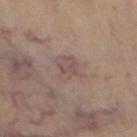{
  "biopsy_status": "not biopsied; imaged during a skin examination",
  "image": {
    "source": "total-body photography crop",
    "field_of_view_mm": 15
  },
  "site": "right thigh",
  "patient": {
    "sex": "female",
    "age_approx": 40
  },
  "lighting": "cross-polarized"
}